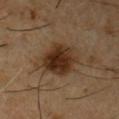biopsy_status: not biopsied; imaged during a skin examination
site: chest
patient:
  sex: male
  age_approx: 55
lighting: cross-polarized
image:
  source: total-body photography crop
  field_of_view_mm: 15